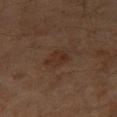Case summary:
• workup — catalogued during a skin exam; not biopsied
• patient — male, about 60 years old
• image — ~15 mm tile from a whole-body skin photo
• site — the right thigh
• TBP lesion metrics — an outline eccentricity of about 0.65 (0 = round, 1 = elongated) and a symmetry-axis asymmetry near 0.4; a mean CIELAB color near L≈26 a*≈16 b*≈22 and a lesion–skin lightness drop of about 5; a nevus-likeness score of about 30/100 and a detector confidence of about 100 out of 100 that the crop contains a lesion
• diameter — ~2.5 mm (longest diameter)